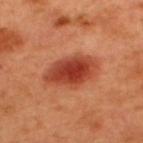The lesion-visualizer software estimated a border-irregularity index near 1.5/10 and internal color variation of about 5 on a 0–10 scale.
On the back.
The subject is a male approximately 50 years of age.
A lesion tile, about 15 mm wide, cut from a 3D total-body photograph.